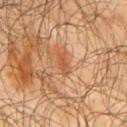Acquisition and patient details:
Cropped from a total-body skin-imaging series; the visible field is about 15 mm. The tile uses cross-polarized illumination. Automated image analysis of the tile measured a footprint of about 3.5 mm² and an outline eccentricity of about 0.85 (0 = round, 1 = elongated). And it measured a lesion color around L≈52 a*≈25 b*≈36 in CIELAB and roughly 8 lightness units darker than nearby skin. It also reported a classifier nevus-likeness of about 75/100 and a detector confidence of about 100 out of 100 that the crop contains a lesion. The subject is a male roughly 65 years of age. From the right upper arm.